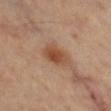Part of a total-body skin-imaging series; this lesion was reviewed on a skin check and was not flagged for biopsy.
Cropped from a total-body skin-imaging series; the visible field is about 15 mm.
This is a cross-polarized tile.
A male patient aged around 60.
An algorithmic analysis of the crop reported a classifier nevus-likeness of about 90/100 and lesion-presence confidence of about 100/100.
From the left thigh.
Longest diameter approximately 3.5 mm.